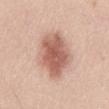follow-up: imaged on a skin check; not biopsied
lesion size: ~6.5 mm (longest diameter)
TBP lesion metrics: roughly 14 lightness units darker than nearby skin and a lesion-to-skin contrast of about 9 (normalized; higher = more distinct); a border-irregularity index near 2/10 and a within-lesion color-variation index near 4.5/10
tile lighting: white-light
subject: male, roughly 25 years of age
location: the abdomen
image source: 15 mm crop, total-body photography